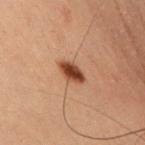  biopsy_status: not biopsied; imaged during a skin examination
  image:
    source: total-body photography crop
    field_of_view_mm: 15
  site: arm
  lesion_size:
    long_diameter_mm_approx: 3.0
  patient:
    sex: male
    age_approx: 60